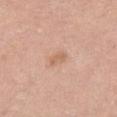Q: Was this lesion biopsied?
A: imaged on a skin check; not biopsied
Q: What is the imaging modality?
A: total-body-photography crop, ~15 mm field of view
Q: Where on the body is the lesion?
A: the right thigh
Q: Who is the patient?
A: female, aged 48 to 52
Q: Lesion size?
A: ≈2.5 mm
Q: What did automated image analysis measure?
A: a border-irregularity rating of about 3.5/10, a color-variation rating of about 0.5/10, and a peripheral color-asymmetry measure near 0